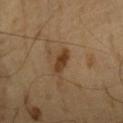The subject is a male aged around 60.
Cropped from a total-body skin-imaging series; the visible field is about 15 mm.
From the chest.